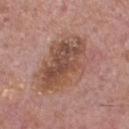Clinical impression: Recorded during total-body skin imaging; not selected for excision or biopsy. Context: The recorded lesion diameter is about 8 mm. A 15 mm close-up extracted from a 3D total-body photography capture. An algorithmic analysis of the crop reported a mean CIELAB color near L≈50 a*≈20 b*≈27, a lesion–skin lightness drop of about 10, and a normalized border contrast of about 7.5. The lesion is located on the chest. This is a white-light tile. The patient is a male aged 73–77.